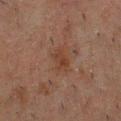| feature | finding |
|---|---|
| workup | no biopsy performed (imaged during a skin exam) |
| automated metrics | a border-irregularity rating of about 2.5/10 and a within-lesion color-variation index near 2.5/10; lesion-presence confidence of about 100/100 |
| patient | male, in their 50s |
| acquisition | ~15 mm tile from a whole-body skin photo |
| lesion size | ~3 mm (longest diameter) |
| anatomic site | the chest |